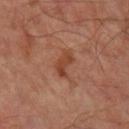The lesion was tiled from a total-body skin photograph and was not biopsied.
Approximately 3 mm at its widest.
A region of skin cropped from a whole-body photographic capture, roughly 15 mm wide.
The lesion is on the right lower leg.
The subject is a male roughly 70 years of age.
Captured under cross-polarized illumination.